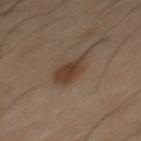Q: Is there a histopathology result?
A: imaged on a skin check; not biopsied
Q: Who is the patient?
A: male, approximately 40 years of age
Q: Where on the body is the lesion?
A: the mid back
Q: What is the imaging modality?
A: 15 mm crop, total-body photography
Q: Automated lesion metrics?
A: an area of roughly 8 mm², an eccentricity of roughly 0.85, and a shape-asymmetry score of about 0.3 (0 = symmetric); a normalized border contrast of about 8; border irregularity of about 3.5 on a 0–10 scale, internal color variation of about 3 on a 0–10 scale, and a peripheral color-asymmetry measure near 1
Q: Illumination type?
A: cross-polarized